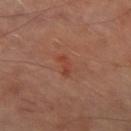biopsy_status: not biopsied; imaged during a skin examination
site: right thigh
lighting: cross-polarized
image:
  source: total-body photography crop
  field_of_view_mm: 15
lesion_size:
  long_diameter_mm_approx: 2.5
patient:
  sex: male
  age_approx: 70